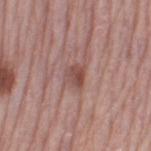| feature | finding |
|---|---|
| patient | female, in their mid- to late 60s |
| image source | ~15 mm tile from a whole-body skin photo |
| anatomic site | the left thigh |
| diameter | ~2.5 mm (longest diameter) |